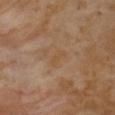A 15 mm close-up tile from a total-body photography series done for melanoma screening. About 2.5 mm across. Located on the chest. A female patient aged approximately 55. This is a cross-polarized tile.